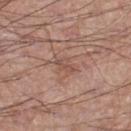• biopsy status: total-body-photography surveillance lesion; no biopsy
• anatomic site: the left thigh
• automated lesion analysis: a symmetry-axis asymmetry near 0.35; border irregularity of about 3.5 on a 0–10 scale, a color-variation rating of about 1/10, and radial color variation of about 0; a nevus-likeness score of about 0/100
• diameter: ≈2.5 mm
• acquisition: ~15 mm tile from a whole-body skin photo
• tile lighting: white-light illumination
• patient: male, roughly 60 years of age